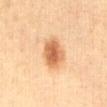Clinical impression: Part of a total-body skin-imaging series; this lesion was reviewed on a skin check and was not flagged for biopsy. Acquisition and patient details: Located on the front of the torso. This is a cross-polarized tile. A lesion tile, about 15 mm wide, cut from a 3D total-body photograph. A female subject, aged approximately 30.